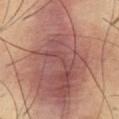- workup: imaged on a skin check; not biopsied
- location: the front of the torso
- acquisition: total-body-photography crop, ~15 mm field of view
- subject: male, aged 53 to 57